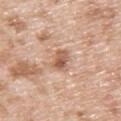biopsy_status: not biopsied; imaged during a skin examination
lesion_size:
  long_diameter_mm_approx: 3.0
patient:
  sex: male
  age_approx: 50
image:
  source: total-body photography crop
  field_of_view_mm: 15
site: upper back
automated_metrics:
  border_irregularity_0_10: 2.5
  color_variation_0_10: 5.5
  peripheral_color_asymmetry: 2.0
  nevus_likeness_0_100: 0
lighting: white-light